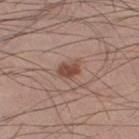<record>
<biopsy_status>not biopsied; imaged during a skin examination</biopsy_status>
<lighting>white-light</lighting>
<patient>
  <sex>male</sex>
  <age_approx>35</age_approx>
</patient>
<image>
  <source>total-body photography crop</source>
  <field_of_view_mm>15</field_of_view_mm>
</image>
<lesion_size>
  <long_diameter_mm_approx>2.5</long_diameter_mm_approx>
</lesion_size>
<site>right lower leg</site>
</record>